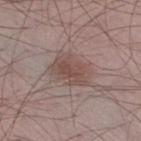Assessment:
This lesion was catalogued during total-body skin photography and was not selected for biopsy.
Image and clinical context:
A lesion tile, about 15 mm wide, cut from a 3D total-body photograph. This is a white-light tile. The lesion's longest dimension is about 4.5 mm. Automated tile analysis of the lesion measured a footprint of about 11 mm² and a shape eccentricity near 0.75. The software also gave a border-irregularity index near 2/10 and internal color variation of about 3 on a 0–10 scale. From the right thigh. A male subject roughly 35 years of age.